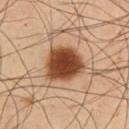Background:
Captured under cross-polarized illumination. A male subject, roughly 55 years of age. The total-body-photography lesion software estimated a lesion color around L≈43 a*≈22 b*≈33 in CIELAB, about 19 CIELAB-L* units darker than the surrounding skin, and a normalized lesion–skin contrast near 14. And it measured border irregularity of about 2 on a 0–10 scale, a color-variation rating of about 4/10, and radial color variation of about 1. And it measured an automated nevus-likeness rating near 100 out of 100 and a detector confidence of about 100 out of 100 that the crop contains a lesion. A 15 mm crop from a total-body photograph taken for skin-cancer surveillance. Longest diameter approximately 5 mm. The lesion is on the front of the torso.